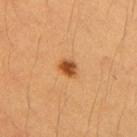Q: Was a biopsy performed?
A: total-body-photography surveillance lesion; no biopsy
Q: Patient demographics?
A: female, aged approximately 40
Q: Where on the body is the lesion?
A: the right upper arm
Q: What is the imaging modality?
A: ~15 mm crop, total-body skin-cancer survey
Q: What did automated image analysis measure?
A: a footprint of about 4 mm², an outline eccentricity of about 0.6 (0 = round, 1 = elongated), and a shape-asymmetry score of about 0.15 (0 = symmetric); a classifier nevus-likeness of about 100/100 and a lesion-detection confidence of about 100/100
Q: Lesion size?
A: about 2.5 mm
Q: What lighting was used for the tile?
A: cross-polarized